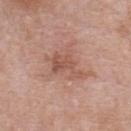workup=no biopsy performed (imaged during a skin exam); location=the chest; image source=~15 mm tile from a whole-body skin photo; tile lighting=white-light; lesion size=~5 mm (longest diameter); subject=male, about 55 years old.